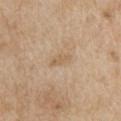workup = no biopsy performed (imaged during a skin exam)
tile lighting = white-light
subject = male, in their 70s
image = ~15 mm tile from a whole-body skin photo
site = the arm
size = about 2.5 mm
automated lesion analysis = a footprint of about 2.5 mm² and two-axis asymmetry of about 0.35